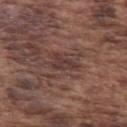Captured during whole-body skin photography for melanoma surveillance; the lesion was not biopsied. Longest diameter approximately 3 mm. The subject is a male aged around 75. Cropped from a total-body skin-imaging series; the visible field is about 15 mm. Imaged with white-light lighting. The lesion is located on the left upper arm.